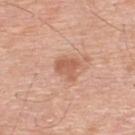This lesion was catalogued during total-body skin photography and was not selected for biopsy.
Longest diameter approximately 3 mm.
This is a white-light tile.
Cropped from a total-body skin-imaging series; the visible field is about 15 mm.
Located on the upper back.
The subject is a male aged around 60.